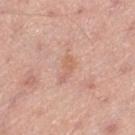Assessment: Captured during whole-body skin photography for melanoma surveillance; the lesion was not biopsied. Clinical summary: A region of skin cropped from a whole-body photographic capture, roughly 15 mm wide. The lesion is on the left thigh. Captured under white-light illumination. A male subject, aged approximately 50.